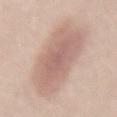Assessment: Imaged during a routine full-body skin examination; the lesion was not biopsied and no histopathology is available. Acquisition and patient details: The lesion's longest dimension is about 9.5 mm. On the lower back. Captured under white-light illumination. A female patient in their mid-40s. A 15 mm crop from a total-body photograph taken for skin-cancer surveillance.